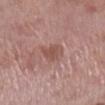Q: Was a biopsy performed?
A: no biopsy performed (imaged during a skin exam)